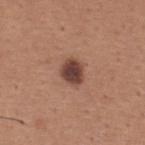The lesion was photographed on a routine skin check and not biopsied; there is no pathology result.
A male subject approximately 65 years of age.
Automated tile analysis of the lesion measured a border-irregularity rating of about 1.5/10 and internal color variation of about 2.5 on a 0–10 scale. The software also gave a nevus-likeness score of about 75/100 and lesion-presence confidence of about 100/100.
The tile uses white-light illumination.
A roughly 15 mm field-of-view crop from a total-body skin photograph.
The lesion's longest dimension is about 3 mm.
On the back.